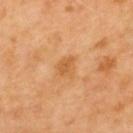| feature | finding |
|---|---|
| biopsy status | imaged on a skin check; not biopsied |
| patient | male, aged 68–72 |
| anatomic site | the upper back |
| image | ~15 mm tile from a whole-body skin photo |